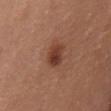| key | value |
|---|---|
| follow-up | catalogued during a skin exam; not biopsied |
| patient | female, aged approximately 65 |
| automated lesion analysis | a normalized border contrast of about 9; an automated nevus-likeness rating near 95 out of 100 and lesion-presence confidence of about 100/100 |
| image source | 15 mm crop, total-body photography |
| tile lighting | white-light |
| lesion size | ~3.5 mm (longest diameter) |
| site | the chest |